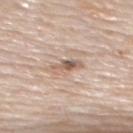- biopsy status — catalogued during a skin exam; not biopsied
- image — total-body-photography crop, ~15 mm field of view
- automated metrics — a footprint of about 5.5 mm², an outline eccentricity of about 0.9 (0 = round, 1 = elongated), and two-axis asymmetry of about 0.25; an average lesion color of about L≈61 a*≈16 b*≈26 (CIELAB) and a normalized lesion–skin contrast near 7; a border-irregularity index near 3/10 and radial color variation of about 4; an automated nevus-likeness rating near 0 out of 100 and a lesion-detection confidence of about 85/100
- subject — female, aged 68 to 72
- lighting — white-light illumination
- diameter — ~4 mm (longest diameter)
- location — the upper back